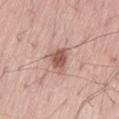biopsy status: no biopsy performed (imaged during a skin exam) | size: ≈3.5 mm | site: the left thigh | subject: male, about 55 years old | acquisition: ~15 mm crop, total-body skin-cancer survey | tile lighting: white-light illumination | TBP lesion metrics: about 13 CIELAB-L* units darker than the surrounding skin; an automated nevus-likeness rating near 90 out of 100.